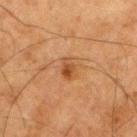Imaged during a routine full-body skin examination; the lesion was not biopsied and no histopathology is available. A 15 mm close-up extracted from a 3D total-body photography capture. The patient is a male approximately 80 years of age. The tile uses cross-polarized illumination. On the leg. Automated image analysis of the tile measured a color-variation rating of about 4.5/10 and peripheral color asymmetry of about 1.5. The software also gave a classifier nevus-likeness of about 80/100 and a lesion-detection confidence of about 100/100. Approximately 2.5 mm at its widest.